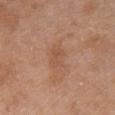{
  "site": "chest",
  "image": {
    "source": "total-body photography crop",
    "field_of_view_mm": 15
  },
  "patient": {
    "sex": "female",
    "age_approx": 30
  }
}